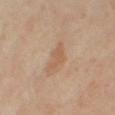Clinical impression: Imaged during a routine full-body skin examination; the lesion was not biopsied and no histopathology is available. Image and clinical context: The lesion is on the right thigh. A 15 mm crop from a total-body photograph taken for skin-cancer surveillance. This is a cross-polarized tile. About 2.5 mm across. A female subject aged around 50.